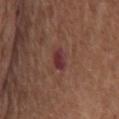Q: Is there a histopathology result?
A: no biopsy performed (imaged during a skin exam)
Q: What kind of image is this?
A: ~15 mm tile from a whole-body skin photo
Q: What are the patient's age and sex?
A: male, about 75 years old
Q: Illumination type?
A: white-light illumination
Q: Lesion size?
A: ~2.5 mm (longest diameter)
Q: Automated lesion metrics?
A: a border-irregularity rating of about 2.5/10, a color-variation rating of about 4/10, and peripheral color asymmetry of about 1.5; an automated nevus-likeness rating near 0 out of 100 and a lesion-detection confidence of about 100/100
Q: Lesion location?
A: the chest